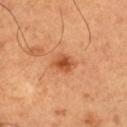Q: Was a biopsy performed?
A: catalogued during a skin exam; not biopsied
Q: Automated lesion metrics?
A: a footprint of about 5 mm², an outline eccentricity of about 0.65 (0 = round, 1 = elongated), and a shape-asymmetry score of about 0.3 (0 = symmetric); a nevus-likeness score of about 80/100
Q: What are the patient's age and sex?
A: male, in their 50s
Q: How was this image acquired?
A: ~15 mm crop, total-body skin-cancer survey
Q: Lesion location?
A: the leg